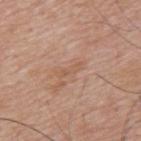Clinical impression: This lesion was catalogued during total-body skin photography and was not selected for biopsy. Context: This is a white-light tile. A male patient roughly 55 years of age. About 3.5 mm across. From the back. This image is a 15 mm lesion crop taken from a total-body photograph. Automated image analysis of the tile measured a lesion area of about 2.5 mm².